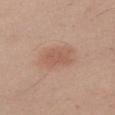Q: Was a biopsy performed?
A: total-body-photography surveillance lesion; no biopsy
Q: Lesion location?
A: the right thigh
Q: How was this image acquired?
A: ~15 mm crop, total-body skin-cancer survey
Q: Lesion size?
A: ≈3.5 mm
Q: Who is the patient?
A: female, about 30 years old